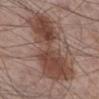Recorded during total-body skin imaging; not selected for excision or biopsy. Longest diameter approximately 10 mm. On the leg. A roughly 15 mm field-of-view crop from a total-body skin photograph. A male patient, roughly 60 years of age. The total-body-photography lesion software estimated a lesion area of about 40 mm², a shape eccentricity near 0.85, and two-axis asymmetry of about 0.35. And it measured an average lesion color of about L≈45 a*≈19 b*≈24 (CIELAB). The software also gave a border-irregularity index near 5.5/10, a color-variation rating of about 5.5/10, and a peripheral color-asymmetry measure near 2.